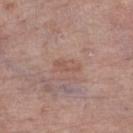* biopsy status: no biopsy performed (imaged during a skin exam)
* subject: female, aged 83 or over
* site: the right lower leg
* image-analysis metrics: a lesion area of about 3.5 mm² and an eccentricity of roughly 0.95; a color-variation rating of about 0.5/10 and peripheral color asymmetry of about 0; a classifier nevus-likeness of about 0/100 and a detector confidence of about 100 out of 100 that the crop contains a lesion
* image: ~15 mm tile from a whole-body skin photo
* illumination: white-light illumination